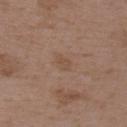Assessment: Captured during whole-body skin photography for melanoma surveillance; the lesion was not biopsied. Acquisition and patient details: A female subject, about 35 years old. A 15 mm close-up tile from a total-body photography series done for melanoma screening. The lesion is on the upper back.